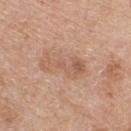Clinical impression: This lesion was catalogued during total-body skin photography and was not selected for biopsy. Image and clinical context: The lesion-visualizer software estimated an area of roughly 9 mm² and an outline eccentricity of about 0.95 (0 = round, 1 = elongated). The software also gave a lesion color around L≈58 a*≈20 b*≈30 in CIELAB and a normalized lesion–skin contrast near 5.5. The lesion is located on the upper back. A lesion tile, about 15 mm wide, cut from a 3D total-body photograph. A male subject aged approximately 60.